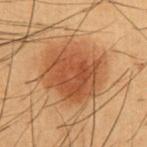Recorded during total-body skin imaging; not selected for excision or biopsy. A 15 mm close-up tile from a total-body photography series done for melanoma screening. Measured at roughly 6.5 mm in maximum diameter. The lesion-visualizer software estimated a color-variation rating of about 4/10 and radial color variation of about 1.5. The software also gave a classifier nevus-likeness of about 95/100 and a lesion-detection confidence of about 100/100. From the abdomen. Imaged with cross-polarized lighting. A male subject, roughly 50 years of age.